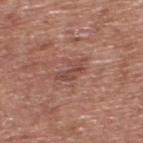workup: no biopsy performed (imaged during a skin exam) | lighting: white-light | TBP lesion metrics: an area of roughly 4.5 mm², a shape eccentricity near 0.9, and a shape-asymmetry score of about 0.45 (0 = symmetric); a mean CIELAB color near L≈47 a*≈23 b*≈25, roughly 8 lightness units darker than nearby skin, and a normalized border contrast of about 6.5; a nevus-likeness score of about 0/100 and lesion-presence confidence of about 95/100 | anatomic site: the upper back | image source: total-body-photography crop, ~15 mm field of view | diameter: ~4 mm (longest diameter) | subject: male, aged 73–77.